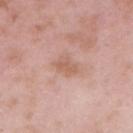This lesion was catalogued during total-body skin photography and was not selected for biopsy. Measured at roughly 3 mm in maximum diameter. Captured under white-light illumination. On the right upper arm. A 15 mm crop from a total-body photograph taken for skin-cancer surveillance. The subject is a male approximately 55 years of age.